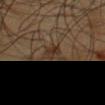The lesion was tiled from a total-body skin photograph and was not biopsied.
Imaged with cross-polarized lighting.
Automated tile analysis of the lesion measured an average lesion color of about L≈29 a*≈13 b*≈25 (CIELAB) and a lesion-to-skin contrast of about 7 (normalized; higher = more distinct). It also reported border irregularity of about 4 on a 0–10 scale, a color-variation rating of about 2.5/10, and a peripheral color-asymmetry measure near 1.
Located on the upper back.
A male subject, in their mid- to late 60s.
This image is a 15 mm lesion crop taken from a total-body photograph.
The recorded lesion diameter is about 2.5 mm.